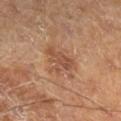Impression:
Captured during whole-body skin photography for melanoma surveillance; the lesion was not biopsied.
Image and clinical context:
Cropped from a whole-body photographic skin survey; the tile spans about 15 mm. The subject is a male approximately 60 years of age. Measured at roughly 3.5 mm in maximum diameter. The total-body-photography lesion software estimated an eccentricity of roughly 0.7 and two-axis asymmetry of about 0.4. The software also gave a mean CIELAB color near L≈47 a*≈21 b*≈30, roughly 8 lightness units darker than nearby skin, and a normalized border contrast of about 6. It also reported an automated nevus-likeness rating near 15 out of 100 and a detector confidence of about 100 out of 100 that the crop contains a lesion. Imaged with cross-polarized lighting. Located on the leg.